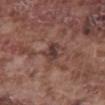biopsy status — total-body-photography surveillance lesion; no biopsy
patient — male, aged approximately 75
acquisition — ~15 mm tile from a whole-body skin photo
tile lighting — white-light
lesion size — ~3 mm (longest diameter)
automated lesion analysis — a lesion area of about 4.5 mm², an eccentricity of roughly 0.7, and two-axis asymmetry of about 0.2; a border-irregularity index near 2/10 and a color-variation rating of about 3.5/10; an automated nevus-likeness rating near 0 out of 100
anatomic site — the abdomen